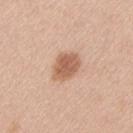No biopsy was performed on this lesion — it was imaged during a full skin examination and was not determined to be concerning. Approximately 4 mm at its widest. A region of skin cropped from a whole-body photographic capture, roughly 15 mm wide. A female patient in their mid-50s. The lesion is located on the lower back. Imaged with white-light lighting.